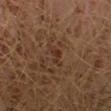Assessment:
This lesion was catalogued during total-body skin photography and was not selected for biopsy.
Image and clinical context:
A region of skin cropped from a whole-body photographic capture, roughly 15 mm wide. Approximately 2.5 mm at its widest. Located on the left lower leg. A male patient approximately 30 years of age. Captured under cross-polarized illumination.